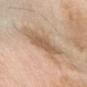- notes — catalogued during a skin exam; not biopsied
- acquisition — total-body-photography crop, ~15 mm field of view
- TBP lesion metrics — a footprint of about 13 mm² and a shape-asymmetry score of about 0.2 (0 = symmetric); a lesion color around L≈60 a*≈16 b*≈32 in CIELAB, a lesion–skin lightness drop of about 10, and a normalized lesion–skin contrast near 6.5; a detector confidence of about 100 out of 100 that the crop contains a lesion
- anatomic site — the right forearm
- subject — male, aged 53 to 57